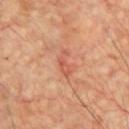Assessment: Part of a total-body skin-imaging series; this lesion was reviewed on a skin check and was not flagged for biopsy. Acquisition and patient details: The lesion is located on the chest. The lesion-visualizer software estimated a lesion area of about 3 mm² and a shape eccentricity near 0.9. The software also gave a border-irregularity index near 6.5/10, a color-variation rating of about 0.5/10, and radial color variation of about 0. Cropped from a total-body skin-imaging series; the visible field is about 15 mm. This is a cross-polarized tile. The patient is a male aged 58 to 62. Approximately 3 mm at its widest.